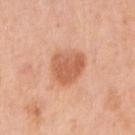No biopsy was performed on this lesion — it was imaged during a full skin examination and was not determined to be concerning. This is a white-light tile. Longest diameter approximately 4 mm. The subject is a female aged approximately 40. A region of skin cropped from a whole-body photographic capture, roughly 15 mm wide. On the right upper arm.